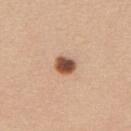Clinical impression:
Part of a total-body skin-imaging series; this lesion was reviewed on a skin check and was not flagged for biopsy.
Image and clinical context:
Located on the upper back. Automated image analysis of the tile measured an area of roughly 5 mm², an eccentricity of roughly 0.65, and two-axis asymmetry of about 0.2. The tile uses white-light illumination. The patient is a female approximately 40 years of age. Cropped from a whole-body photographic skin survey; the tile spans about 15 mm.